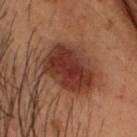Notes:
– workup · imaged on a skin check; not biopsied
– image · ~15 mm tile from a whole-body skin photo
– patient · male, in their mid-50s
– tile lighting · cross-polarized
– location · the head or neck